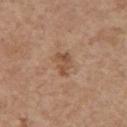workup: total-body-photography surveillance lesion; no biopsy
patient: female, aged approximately 65
image source: total-body-photography crop, ~15 mm field of view
anatomic site: the right upper arm